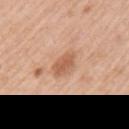Part of a total-body skin-imaging series; this lesion was reviewed on a skin check and was not flagged for biopsy. The lesion-visualizer software estimated a lesion area of about 5.5 mm², a shape eccentricity near 0.85, and a symmetry-axis asymmetry near 0.2. The analysis additionally found border irregularity of about 2 on a 0–10 scale and a color-variation rating of about 2.5/10. On the left upper arm. A lesion tile, about 15 mm wide, cut from a 3D total-body photograph. The lesion's longest dimension is about 3.5 mm. A male patient, about 55 years old.